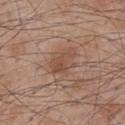Q: Was this lesion biopsied?
A: total-body-photography surveillance lesion; no biopsy
Q: What is the anatomic site?
A: the chest
Q: Who is the patient?
A: male, approximately 60 years of age
Q: What is the imaging modality?
A: 15 mm crop, total-body photography
Q: Illumination type?
A: white-light
Q: How large is the lesion?
A: ~4 mm (longest diameter)
Q: What did automated image analysis measure?
A: a lesion area of about 6 mm², a shape eccentricity near 0.85, and two-axis asymmetry of about 0.45; a border-irregularity rating of about 4.5/10 and a within-lesion color-variation index near 2/10